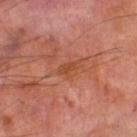The lesion was tiled from a total-body skin photograph and was not biopsied.
Longest diameter approximately 2.5 mm.
The lesion is located on the left thigh.
The subject is a male about 70 years old.
The total-body-photography lesion software estimated a border-irregularity rating of about 3.5/10, a color-variation rating of about 0.5/10, and a peripheral color-asymmetry measure near 0.
This is a cross-polarized tile.
Cropped from a whole-body photographic skin survey; the tile spans about 15 mm.